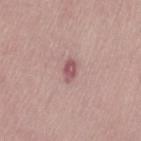Recorded during total-body skin imaging; not selected for excision or biopsy. Captured under white-light illumination. A female patient, about 50 years old. A 15 mm close-up tile from a total-body photography series done for melanoma screening. From the lower back. The lesion's longest dimension is about 2.5 mm. The total-body-photography lesion software estimated a mean CIELAB color near L≈54 a*≈25 b*≈17 and about 12 CIELAB-L* units darker than the surrounding skin. And it measured a classifier nevus-likeness of about 0/100 and a detector confidence of about 100 out of 100 that the crop contains a lesion.